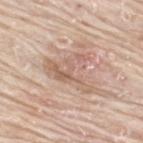<case>
  <biopsy_status>not biopsied; imaged during a skin examination</biopsy_status>
  <lighting>white-light</lighting>
  <site>upper back</site>
  <lesion_size>
    <long_diameter_mm_approx>6.5</long_diameter_mm_approx>
  </lesion_size>
  <automated_metrics>
    <area_mm2_approx>14.0</area_mm2_approx>
    <eccentricity>0.7</eccentricity>
    <shape_asymmetry>0.7</shape_asymmetry>
    <color_variation_0_10>6.0</color_variation_0_10>
    <peripheral_color_asymmetry>2.0</peripheral_color_asymmetry>
  </automated_metrics>
  <patient>
    <sex>male</sex>
    <age_approx>75</age_approx>
  </patient>
  <image>
    <source>total-body photography crop</source>
    <field_of_view_mm>15</field_of_view_mm>
  </image>
</case>